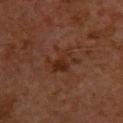follow-up = total-body-photography surveillance lesion; no biopsy | illumination = cross-polarized | location = the chest | image = ~15 mm crop, total-body skin-cancer survey | patient = male, in their 60s.